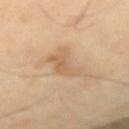biopsy status = total-body-photography surveillance lesion; no biopsy
lighting = cross-polarized illumination
patient = male, aged around 65
imaging modality = total-body-photography crop, ~15 mm field of view
body site = the right forearm
automated lesion analysis = lesion-presence confidence of about 100/100
lesion diameter = ~5 mm (longest diameter)